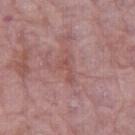location: the right thigh; image: ~15 mm crop, total-body skin-cancer survey; patient: female, aged 63 to 67.